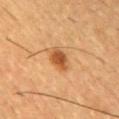Q: Was a biopsy performed?
A: catalogued during a skin exam; not biopsied
Q: What are the patient's age and sex?
A: male, in their mid- to late 50s
Q: What did automated image analysis measure?
A: a footprint of about 5 mm², an eccentricity of roughly 0.75, and a symmetry-axis asymmetry near 0.25; a border-irregularity rating of about 2.5/10, internal color variation of about 3 on a 0–10 scale, and radial color variation of about 1; an automated nevus-likeness rating near 95 out of 100 and lesion-presence confidence of about 100/100
Q: What is the anatomic site?
A: the upper back
Q: How was this image acquired?
A: ~15 mm tile from a whole-body skin photo